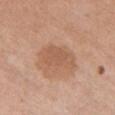Captured during whole-body skin photography for melanoma surveillance; the lesion was not biopsied. The lesion is on the chest. A female patient, aged 53 to 57. The tile uses white-light illumination. Cropped from a total-body skin-imaging series; the visible field is about 15 mm.